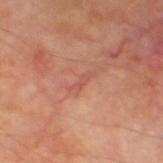<lesion>
  <biopsy_status>not biopsied; imaged during a skin examination</biopsy_status>
  <lesion_size>
    <long_diameter_mm_approx>3.0</long_diameter_mm_approx>
  </lesion_size>
  <site>leg</site>
  <patient>
    <sex>male</sex>
    <age_approx>70</age_approx>
  </patient>
  <image>
    <source>total-body photography crop</source>
    <field_of_view_mm>15</field_of_view_mm>
  </image>
</lesion>